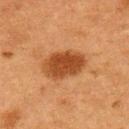Recorded during total-body skin imaging; not selected for excision or biopsy. This is a cross-polarized tile. An algorithmic analysis of the crop reported border irregularity of about 1.5 on a 0–10 scale and radial color variation of about 1. The software also gave a detector confidence of about 100 out of 100 that the crop contains a lesion. Cropped from a total-body skin-imaging series; the visible field is about 15 mm. From the left upper arm. A male subject, aged approximately 75.